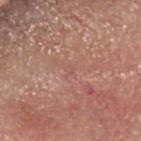Assessment: Part of a total-body skin-imaging series; this lesion was reviewed on a skin check and was not flagged for biopsy. Acquisition and patient details: The patient is a male about 80 years old. A 15 mm close-up tile from a total-body photography series done for melanoma screening. The lesion is on the head or neck. Imaged with white-light lighting. Approximately 1 mm at its widest.